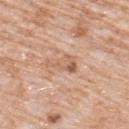follow-up=catalogued during a skin exam; not biopsied | site=the upper back | acquisition=total-body-photography crop, ~15 mm field of view | lesion size=≈3.5 mm | illumination=white-light illumination | subject=male, roughly 80 years of age.